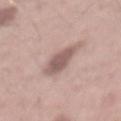{
  "biopsy_status": "not biopsied; imaged during a skin examination",
  "patient": {
    "sex": "male",
    "age_approx": 55
  },
  "image": {
    "source": "total-body photography crop",
    "field_of_view_mm": 15
  },
  "site": "back",
  "lesion_size": {
    "long_diameter_mm_approx": 5.5
  },
  "lighting": "white-light",
  "automated_metrics": {
    "area_mm2_approx": 9.0,
    "shape_asymmetry": 0.3,
    "cielab_L": 57,
    "cielab_a": 17,
    "cielab_b": 21,
    "vs_skin_darker_L": 12.0,
    "vs_skin_contrast_norm": 8.0,
    "border_irregularity_0_10": 3.5,
    "color_variation_0_10": 3.0,
    "peripheral_color_asymmetry": 1.0
  }
}